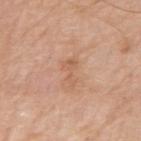• biopsy status: no biopsy performed (imaged during a skin exam)
• tile lighting: white-light
• subject: male, aged 78 to 82
• image: total-body-photography crop, ~15 mm field of view
• anatomic site: the right upper arm
• lesion size: ≈3.5 mm